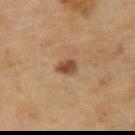Impression:
Part of a total-body skin-imaging series; this lesion was reviewed on a skin check and was not flagged for biopsy.
Image and clinical context:
Captured under cross-polarized illumination. The patient is a female aged approximately 60. The recorded lesion diameter is about 2.5 mm. Automated image analysis of the tile measured a normalized lesion–skin contrast near 9. The software also gave an automated nevus-likeness rating near 90 out of 100 and a detector confidence of about 100 out of 100 that the crop contains a lesion. On the upper back. A lesion tile, about 15 mm wide, cut from a 3D total-body photograph.